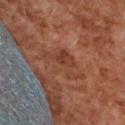Clinical impression:
No biopsy was performed on this lesion — it was imaged during a full skin examination and was not determined to be concerning.
Image and clinical context:
The lesion is on the upper back. The patient is a female roughly 60 years of age. A region of skin cropped from a whole-body photographic capture, roughly 15 mm wide.